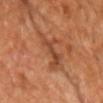Image and clinical context: The subject is a male aged 68 to 72. Approximately 6.5 mm at its widest. Imaged with cross-polarized lighting. On the chest. Automated image analysis of the tile measured a classifier nevus-likeness of about 0/100. A region of skin cropped from a whole-body photographic capture, roughly 15 mm wide.